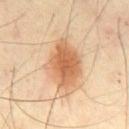| feature | finding |
|---|---|
| follow-up | no biopsy performed (imaged during a skin exam) |
| body site | the abdomen |
| subject | male, about 65 years old |
| TBP lesion metrics | border irregularity of about 2 on a 0–10 scale, a color-variation rating of about 4.5/10, and peripheral color asymmetry of about 1; a nevus-likeness score of about 100/100 and a lesion-detection confidence of about 100/100 |
| acquisition | total-body-photography crop, ~15 mm field of view |
| lighting | cross-polarized |
| size | about 6 mm |